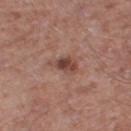Part of a total-body skin-imaging series; this lesion was reviewed on a skin check and was not flagged for biopsy. The lesion-visualizer software estimated a lesion color around L≈44 a*≈21 b*≈25 in CIELAB and about 11 CIELAB-L* units darker than the surrounding skin. And it measured a border-irregularity index near 3.5/10. A roughly 15 mm field-of-view crop from a total-body skin photograph. The lesion is on the leg. The recorded lesion diameter is about 3.5 mm. Captured under white-light illumination. A male subject, aged around 60.